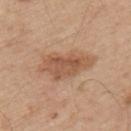No biopsy was performed on this lesion — it was imaged during a full skin examination and was not determined to be concerning. The lesion is located on the back. A roughly 15 mm field-of-view crop from a total-body skin photograph. About 6.5 mm across. The patient is a male about 55 years old. This is a white-light tile.